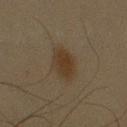Clinical impression:
This lesion was catalogued during total-body skin photography and was not selected for biopsy.
Background:
A 15 mm close-up extracted from a 3D total-body photography capture. Automated image analysis of the tile measured a color-variation rating of about 2/10 and radial color variation of about 0.5. And it measured a nevus-likeness score of about 80/100 and lesion-presence confidence of about 100/100. This is a cross-polarized tile. A male patient approximately 45 years of age. The lesion is located on the right upper arm. Measured at roughly 4 mm in maximum diameter.